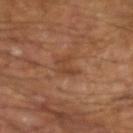The lesion was photographed on a routine skin check and not biopsied; there is no pathology result.
A region of skin cropped from a whole-body photographic capture, roughly 15 mm wide.
A male subject, aged 63–67.
Automated image analysis of the tile measured a border-irregularity rating of about 4.5/10, internal color variation of about 2.5 on a 0–10 scale, and a peripheral color-asymmetry measure near 1. The software also gave a nevus-likeness score of about 0/100 and lesion-presence confidence of about 100/100.
On the left forearm.
Captured under cross-polarized illumination.